The recorded lesion diameter is about 4 mm · on the mid back · captured under white-light illumination · the patient is a female in their 30s · a close-up tile cropped from a whole-body skin photograph, about 15 mm across.
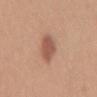| feature | finding |
|---|---|
| histopathology | a dysplastic (Clark) nevus — a benign lesion |